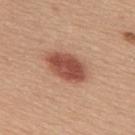workup: imaged on a skin check; not biopsied
lighting: white-light
image: ~15 mm crop, total-body skin-cancer survey
automated metrics: a lesion color around L≈51 a*≈26 b*≈29 in CIELAB, about 15 CIELAB-L* units darker than the surrounding skin, and a lesion-to-skin contrast of about 10 (normalized; higher = more distinct); a classifier nevus-likeness of about 100/100 and lesion-presence confidence of about 100/100
diameter: ~5.5 mm (longest diameter)
body site: the upper back
patient: female, aged 53–57